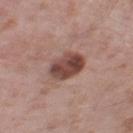Impression:
The lesion was tiled from a total-body skin photograph and was not biopsied.
Image and clinical context:
A male patient, approximately 60 years of age. The lesion is on the leg. Imaged with white-light lighting. A 15 mm close-up extracted from a 3D total-body photography capture.